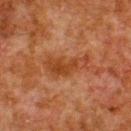Case summary:
- workup — no biopsy performed (imaged during a skin exam)
- lesion diameter — ~5.5 mm (longest diameter)
- image — 15 mm crop, total-body photography
- illumination — cross-polarized illumination
- image-analysis metrics — a footprint of about 9 mm², an eccentricity of roughly 0.95, and a shape-asymmetry score of about 0.5 (0 = symmetric); a border-irregularity index near 6.5/10 and peripheral color asymmetry of about 1; a lesion-detection confidence of about 100/100
- subject — male, aged approximately 80
- anatomic site — the upper back